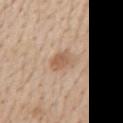Captured during whole-body skin photography for melanoma surveillance; the lesion was not biopsied.